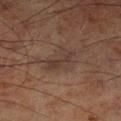Clinical impression: Recorded during total-body skin imaging; not selected for excision or biopsy. Image and clinical context: On the left lower leg. A male patient, aged 68–72. This image is a 15 mm lesion crop taken from a total-body photograph.